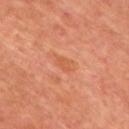<record>
  <biopsy_status>not biopsied; imaged during a skin examination</biopsy_status>
  <image>
    <source>total-body photography crop</source>
    <field_of_view_mm>15</field_of_view_mm>
  </image>
  <lighting>cross-polarized</lighting>
  <lesion_size>
    <long_diameter_mm_approx>2.5</long_diameter_mm_approx>
  </lesion_size>
  <automated_metrics>
    <area_mm2_approx>3.5</area_mm2_approx>
    <eccentricity>0.8</eccentricity>
    <shape_asymmetry>0.2</shape_asymmetry>
    <cielab_L>57</cielab_L>
    <cielab_a>29</cielab_a>
    <cielab_b>38</cielab_b>
    <vs_skin_darker_L>6.0</vs_skin_darker_L>
    <border_irregularity_0_10>2.0</border_irregularity_0_10>
    <peripheral_color_asymmetry>1.0</peripheral_color_asymmetry>
  </automated_metrics>
  <site>front of the torso</site>
  <patient>
    <sex>male</sex>
    <age_approx>50</age_approx>
  </patient>
</record>